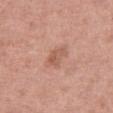{"biopsy_status": "not biopsied; imaged during a skin examination", "lighting": "white-light", "site": "right thigh", "lesion_size": {"long_diameter_mm_approx": 3.0}, "image": {"source": "total-body photography crop", "field_of_view_mm": 15}, "patient": {"sex": "female", "age_approx": 70}}